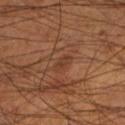Q: Is there a histopathology result?
A: no biopsy performed (imaged during a skin exam)
Q: What kind of image is this?
A: ~15 mm crop, total-body skin-cancer survey
Q: What is the anatomic site?
A: the leg
Q: Who is the patient?
A: male, roughly 55 years of age